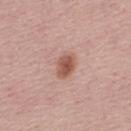| field | value |
|---|---|
| notes | total-body-photography surveillance lesion; no biopsy |
| imaging modality | ~15 mm tile from a whole-body skin photo |
| lesion size | ≈3 mm |
| illumination | white-light illumination |
| subject | male, about 30 years old |